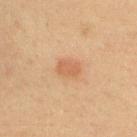<tbp_lesion>
  <biopsy_status>not biopsied; imaged during a skin examination</biopsy_status>
  <site>upper back</site>
  <image>
    <source>total-body photography crop</source>
    <field_of_view_mm>15</field_of_view_mm>
  </image>
  <patient>
    <sex>male</sex>
    <age_approx>40</age_approx>
  </patient>
</tbp_lesion>